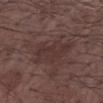The tile uses white-light illumination. A male subject approximately 70 years of age. A close-up tile cropped from a whole-body skin photograph, about 15 mm across. The recorded lesion diameter is about 4.5 mm. The lesion is located on the right upper arm.